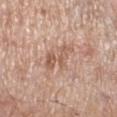Imaged during a routine full-body skin examination; the lesion was not biopsied and no histopathology is available. A region of skin cropped from a whole-body photographic capture, roughly 15 mm wide. This is a white-light tile. A male patient aged around 80. Approximately 5 mm at its widest. On the right lower leg.